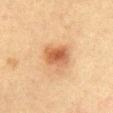| feature | finding |
|---|---|
| subject | female, in their 40s |
| image source | ~15 mm tile from a whole-body skin photo |
| tile lighting | cross-polarized |
| location | the front of the torso |
| diameter | ~3.5 mm (longest diameter) |